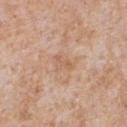notes — imaged on a skin check; not biopsied | image source — 15 mm crop, total-body photography | anatomic site — the chest | patient — male, in their mid-60s | lesion size — ~2.5 mm (longest diameter) | illumination — white-light.